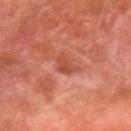• size · ~2.5 mm (longest diameter)
• acquisition · total-body-photography crop, ~15 mm field of view
• automated metrics · a footprint of about 3.5 mm², an outline eccentricity of about 0.8 (0 = round, 1 = elongated), and two-axis asymmetry of about 0.4; a within-lesion color-variation index near 1.5/10 and a peripheral color-asymmetry measure near 0.5; a classifier nevus-likeness of about 0/100
• anatomic site · the left forearm
• subject · male, aged around 80